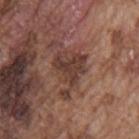Notes:
* workup · imaged on a skin check; not biopsied
* imaging modality · ~15 mm crop, total-body skin-cancer survey
* illumination · white-light
* location · the back
* lesion size · ≈5 mm
* image-analysis metrics · an area of roughly 13 mm², an outline eccentricity of about 0.7 (0 = round, 1 = elongated), and a shape-asymmetry score of about 0.5 (0 = symmetric); an automated nevus-likeness rating near 5 out of 100 and lesion-presence confidence of about 100/100
* patient · male, about 75 years old